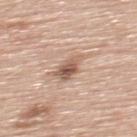Case summary:
• biopsy status · catalogued during a skin exam; not biopsied
• size · about 3 mm
• anatomic site · the back
• acquisition · 15 mm crop, total-body photography
• illumination · white-light illumination
• patient · male, roughly 60 years of age